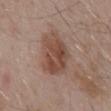Assessment:
Imaged during a routine full-body skin examination; the lesion was not biopsied and no histopathology is available.
Acquisition and patient details:
A 15 mm crop from a total-body photograph taken for skin-cancer surveillance. On the mid back. The patient is a male roughly 55 years of age. Measured at roughly 6 mm in maximum diameter.